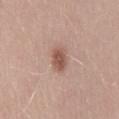<record>
  <biopsy_status>not biopsied; imaged during a skin examination</biopsy_status>
  <patient>
    <sex>male</sex>
    <age_approx>30</age_approx>
  </patient>
  <automated_metrics>
    <cielab_L>54</cielab_L>
    <cielab_a>21</cielab_a>
    <cielab_b>26</cielab_b>
    <vs_skin_darker_L>11.0</vs_skin_darker_L>
    <vs_skin_contrast_norm>8.0</vs_skin_contrast_norm>
    <border_irregularity_0_10>1.5</border_irregularity_0_10>
    <color_variation_0_10>3.0</color_variation_0_10>
    <peripheral_color_asymmetry>1.0</peripheral_color_asymmetry>
    <lesion_detection_confidence_0_100>100</lesion_detection_confidence_0_100>
  </automated_metrics>
  <image>
    <source>total-body photography crop</source>
    <field_of_view_mm>15</field_of_view_mm>
  </image>
  <lighting>white-light</lighting>
</record>